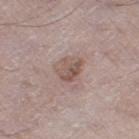Impression:
Captured during whole-body skin photography for melanoma surveillance; the lesion was not biopsied.
Context:
On the left thigh. A 15 mm close-up extracted from a 3D total-body photography capture. The patient is a male aged 63–67.